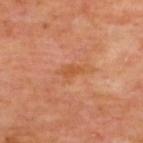Automated image analysis of the tile measured an average lesion color of about L≈52 a*≈26 b*≈39 (CIELAB), a lesion–skin lightness drop of about 6, and a lesion-to-skin contrast of about 6 (normalized; higher = more distinct). The software also gave an automated nevus-likeness rating near 0 out of 100 and lesion-presence confidence of about 100/100. The lesion is on the upper back. The lesion's longest dimension is about 2.5 mm. Captured under cross-polarized illumination. A 15 mm crop from a total-body photograph taken for skin-cancer surveillance. A male patient, in their mid- to late 60s.